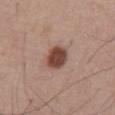Findings:
- subject: male, in their mid-50s
- anatomic site: the abdomen
- acquisition: 15 mm crop, total-body photography
- lesion diameter: about 3 mm
- lighting: white-light
- TBP lesion metrics: a lesion area of about 7 mm² and a shape eccentricity near 0.45; a color-variation rating of about 2.5/10 and radial color variation of about 1